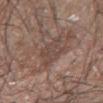Part of a total-body skin-imaging series; this lesion was reviewed on a skin check and was not flagged for biopsy.
Automated image analysis of the tile measured a border-irregularity rating of about 3.5/10 and peripheral color asymmetry of about 1. And it measured a nevus-likeness score of about 0/100 and a lesion-detection confidence of about 90/100.
The subject is a male aged around 65.
The lesion's longest dimension is about 4 mm.
Located on the right forearm.
A close-up tile cropped from a whole-body skin photograph, about 15 mm across.
The tile uses white-light illumination.